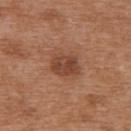Findings:
- workup — total-body-photography surveillance lesion; no biopsy
- image source — total-body-photography crop, ~15 mm field of view
- anatomic site — the back
- patient — female, aged around 40